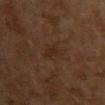  biopsy_status: not biopsied; imaged during a skin examination
  patient:
    sex: female
    age_approx: 60
  site: front of the torso
  automated_metrics:
    cielab_L: 22
    cielab_a: 15
    cielab_b: 22
    vs_skin_darker_L: 4.0
    vs_skin_contrast_norm: 5.5
    border_irregularity_0_10: 3.5
  lesion_size:
    long_diameter_mm_approx: 3.0
  lighting: cross-polarized
  image:
    source: total-body photography crop
    field_of_view_mm: 15